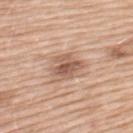Part of a total-body skin-imaging series; this lesion was reviewed on a skin check and was not flagged for biopsy. The tile uses white-light illumination. The recorded lesion diameter is about 4 mm. A female subject in their mid- to late 50s. The lesion is located on the upper back. An algorithmic analysis of the crop reported a lesion area of about 8.5 mm² and a shape-asymmetry score of about 0.3 (0 = symmetric). The analysis additionally found a border-irregularity index near 4/10, internal color variation of about 4.5 on a 0–10 scale, and a peripheral color-asymmetry measure near 1.5. It also reported a nevus-likeness score of about 70/100 and a lesion-detection confidence of about 100/100. A 15 mm close-up tile from a total-body photography series done for melanoma screening.